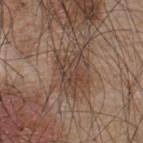This lesion was catalogued during total-body skin photography and was not selected for biopsy. A male patient, in their mid-40s. This image is a 15 mm lesion crop taken from a total-body photograph. The lesion is on the upper back.